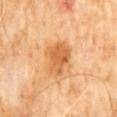The lesion was photographed on a routine skin check and not biopsied; there is no pathology result. A male subject about 60 years old. On the abdomen. Automated image analysis of the tile measured an area of roughly 11 mm², a shape eccentricity near 0.7, and a symmetry-axis asymmetry near 0.25. It also reported a lesion color around L≈60 a*≈25 b*≈43 in CIELAB and a lesion–skin lightness drop of about 11. The software also gave an automated nevus-likeness rating near 90 out of 100. The recorded lesion diameter is about 4.5 mm. A 15 mm close-up tile from a total-body photography series done for melanoma screening. The tile uses cross-polarized illumination.